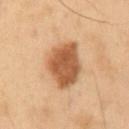notes: no biopsy performed (imaged during a skin exam)
automated metrics: a mean CIELAB color near L≈45 a*≈19 b*≈31, roughly 13 lightness units darker than nearby skin, and a normalized lesion–skin contrast near 10; an automated nevus-likeness rating near 95 out of 100 and lesion-presence confidence of about 100/100
body site: the mid back
patient: male, aged 53 to 57
size: about 6 mm
acquisition: ~15 mm crop, total-body skin-cancer survey
illumination: cross-polarized